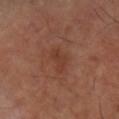Q: Was a biopsy performed?
A: imaged on a skin check; not biopsied
Q: What is the imaging modality?
A: ~15 mm tile from a whole-body skin photo
Q: What is the anatomic site?
A: the arm
Q: Lesion size?
A: ~3 mm (longest diameter)